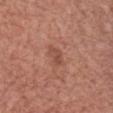biopsy_status: not biopsied; imaged during a skin examination
patient:
  sex: male
  age_approx: 75
automated_metrics:
  area_mm2_approx: 3.0
  eccentricity: 0.85
  shape_asymmetry: 0.25
  nevus_likeness_0_100: 0
  lesion_detection_confidence_0_100: 100
image:
  source: total-body photography crop
  field_of_view_mm: 15
site: chest